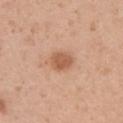The lesion was photographed on a routine skin check and not biopsied; there is no pathology result. A female patient, in their 40s. The tile uses white-light illumination. Located on the left upper arm. A lesion tile, about 15 mm wide, cut from a 3D total-body photograph. Longest diameter approximately 3 mm.